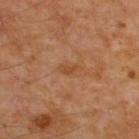Imaged during a routine full-body skin examination; the lesion was not biopsied and no histopathology is available. From the upper back. The subject is a male about 55 years old. A lesion tile, about 15 mm wide, cut from a 3D total-body photograph.